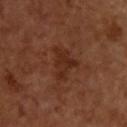Imaged with cross-polarized lighting. A 15 mm close-up extracted from a 3D total-body photography capture. The lesion is on the upper back. Automated image analysis of the tile measured a within-lesion color-variation index near 2/10 and peripheral color asymmetry of about 0.5. The analysis additionally found a nevus-likeness score of about 0/100. Longest diameter approximately 4.5 mm.